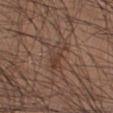workup = no biopsy performed (imaged during a skin exam)
automated lesion analysis = a border-irregularity rating of about 4/10 and internal color variation of about 4.5 on a 0–10 scale; lesion-presence confidence of about 90/100
patient = male, aged around 35
diameter = ~3 mm (longest diameter)
anatomic site = the right lower leg
acquisition = ~15 mm crop, total-body skin-cancer survey
tile lighting = white-light